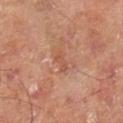Findings:
- anatomic site: the right lower leg
- image: total-body-photography crop, ~15 mm field of view